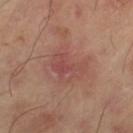Impression:
The lesion was photographed on a routine skin check and not biopsied; there is no pathology result.
Image and clinical context:
Cropped from a whole-body photographic skin survey; the tile spans about 15 mm. From the left thigh.